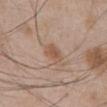Recorded during total-body skin imaging; not selected for excision or biopsy. Located on the abdomen. A region of skin cropped from a whole-body photographic capture, roughly 15 mm wide. Imaged with white-light lighting. An algorithmic analysis of the crop reported an automated nevus-likeness rating near 65 out of 100. Approximately 2.5 mm at its widest. A male patient, in their mid-40s.